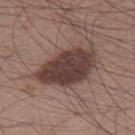<lesion>
<biopsy_status>not biopsied; imaged during a skin examination</biopsy_status>
</lesion>